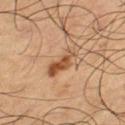workup = catalogued during a skin exam; not biopsied | site = the left thigh | diameter = ≈5 mm | subject = male, aged approximately 65 | image = ~15 mm tile from a whole-body skin photo.